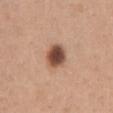• biopsy status — imaged on a skin check; not biopsied
• anatomic site — the abdomen
• image source — ~15 mm tile from a whole-body skin photo
• subject — female, in their 30s